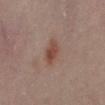The lesion was tiled from a total-body skin photograph and was not biopsied. The lesion is located on the abdomen. This image is a 15 mm lesion crop taken from a total-body photograph. A female subject aged around 40.